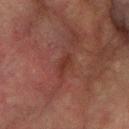The lesion was photographed on a routine skin check and not biopsied; there is no pathology result.
From the arm.
A lesion tile, about 15 mm wide, cut from a 3D total-body photograph.
Approximately 3 mm at its widest.
Automated image analysis of the tile measured a lesion area of about 3 mm², an outline eccentricity of about 0.9 (0 = round, 1 = elongated), and a shape-asymmetry score of about 0.3 (0 = symmetric). The analysis additionally found a border-irregularity rating of about 3/10, internal color variation of about 1 on a 0–10 scale, and radial color variation of about 0.5.
This is a cross-polarized tile.
The subject is a male aged around 75.